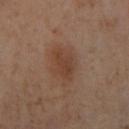Captured under cross-polarized illumination. Automated tile analysis of the lesion measured an outline eccentricity of about 0.8 (0 = round, 1 = elongated) and a shape-asymmetry score of about 0.2 (0 = symmetric). About 4 mm across. A roughly 15 mm field-of-view crop from a total-body skin photograph. On the right lower leg. The subject is a female roughly 55 years of age.